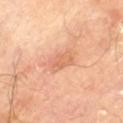notes: imaged on a skin check; not biopsied
acquisition: ~15 mm crop, total-body skin-cancer survey
anatomic site: the left thigh
size: ≈2.5 mm
patient: male, approximately 70 years of age